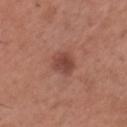automated lesion analysis: a lesion–skin lightness drop of about 10 and a normalized lesion–skin contrast near 7.5 | lesion diameter: ~3.5 mm (longest diameter) | image source: ~15 mm crop, total-body skin-cancer survey | subject: male, roughly 35 years of age | site: the head or neck.